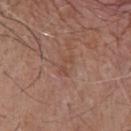The lesion was tiled from a total-body skin photograph and was not biopsied.
A close-up tile cropped from a whole-body skin photograph, about 15 mm across.
Captured under white-light illumination.
The subject is a male aged 63 to 67.
The lesion is located on the chest.
Measured at roughly 2.5 mm in maximum diameter.